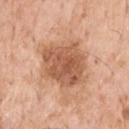Findings:
* imaging modality · ~15 mm tile from a whole-body skin photo
* patient · male, aged approximately 70
* site · the upper back